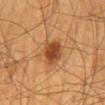The lesion was tiled from a total-body skin photograph and was not biopsied. The tile uses cross-polarized illumination. Longest diameter approximately 3 mm. The subject is a male in their mid-60s. On the mid back. A roughly 15 mm field-of-view crop from a total-body skin photograph. The lesion-visualizer software estimated an area of roughly 7 mm², an outline eccentricity of about 0.2 (0 = round, 1 = elongated), and two-axis asymmetry of about 0.2. The software also gave an average lesion color of about L≈37 a*≈22 b*≈32 (CIELAB), roughly 11 lightness units darker than nearby skin, and a normalized border contrast of about 9.5. And it measured a color-variation rating of about 3/10 and radial color variation of about 1.